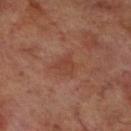The lesion was tiled from a total-body skin photograph and was not biopsied. The lesion is located on the right lower leg. Cropped from a whole-body photographic skin survey; the tile spans about 15 mm. The subject is a male aged 68–72.